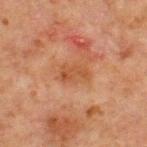Recorded during total-body skin imaging; not selected for excision or biopsy.
A male patient approximately 65 years of age.
Longest diameter approximately 3 mm.
Cropped from a whole-body photographic skin survey; the tile spans about 15 mm.
The total-body-photography lesion software estimated a lesion area of about 4.5 mm², a shape eccentricity near 0.85, and two-axis asymmetry of about 0.45. And it measured a mean CIELAB color near L≈41 a*≈21 b*≈32 and a normalized border contrast of about 6.5. And it measured a classifier nevus-likeness of about 0/100 and a detector confidence of about 100 out of 100 that the crop contains a lesion.
Located on the chest.
This is a cross-polarized tile.